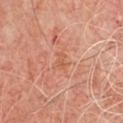Imaged during a routine full-body skin examination; the lesion was not biopsied and no histopathology is available.
From the chest.
About 2 mm across.
The subject is a male about 50 years old.
This is a cross-polarized tile.
A lesion tile, about 15 mm wide, cut from a 3D total-body photograph.
An algorithmic analysis of the crop reported an outline eccentricity of about 0.85 (0 = round, 1 = elongated). The software also gave roughly 6 lightness units darker than nearby skin and a normalized border contrast of about 5. And it measured a nevus-likeness score of about 0/100.